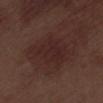Impression:
Recorded during total-body skin imaging; not selected for excision or biopsy.
Image and clinical context:
The lesion-visualizer software estimated a footprint of about 17 mm², an outline eccentricity of about 0.7 (0 = round, 1 = elongated), and a symmetry-axis asymmetry near 0.3. And it measured a mean CIELAB color near L≈25 a*≈19 b*≈18 and about 5 CIELAB-L* units darker than the surrounding skin. The analysis additionally found an automated nevus-likeness rating near 5 out of 100 and lesion-presence confidence of about 100/100. This is a white-light tile. Measured at roughly 5.5 mm in maximum diameter. A region of skin cropped from a whole-body photographic capture, roughly 15 mm wide. A male subject, aged 68–72. Located on the left lower leg.